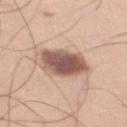Imaged during a routine full-body skin examination; the lesion was not biopsied and no histopathology is available.
Imaged with white-light lighting.
The lesion's longest dimension is about 5.5 mm.
The lesion is located on the leg.
A male patient in their mid- to late 30s.
A region of skin cropped from a whole-body photographic capture, roughly 15 mm wide.